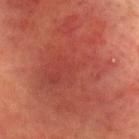Q: Was a biopsy performed?
A: total-body-photography surveillance lesion; no biopsy
Q: What are the patient's age and sex?
A: male, aged 58 to 62
Q: What did automated image analysis measure?
A: an area of roughly 65 mm² and two-axis asymmetry of about 0.25; a border-irregularity rating of about 6.5/10, internal color variation of about 5 on a 0–10 scale, and radial color variation of about 1.5; a nevus-likeness score of about 0/100 and a detector confidence of about 95 out of 100 that the crop contains a lesion
Q: Illumination type?
A: cross-polarized
Q: How large is the lesion?
A: ~12 mm (longest diameter)
Q: How was this image acquired?
A: ~15 mm tile from a whole-body skin photo
Q: Lesion location?
A: the head or neck